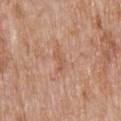Q: Is there a histopathology result?
A: no biopsy performed (imaged during a skin exam)
Q: What kind of image is this?
A: 15 mm crop, total-body photography
Q: Who is the patient?
A: male, aged 78–82
Q: What is the anatomic site?
A: the back